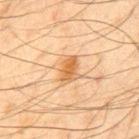| feature | finding |
|---|---|
| follow-up | no biopsy performed (imaged during a skin exam) |
| illumination | cross-polarized illumination |
| patient | male, aged approximately 45 |
| image-analysis metrics | border irregularity of about 3 on a 0–10 scale; a nevus-likeness score of about 90/100 and a detector confidence of about 100 out of 100 that the crop contains a lesion |
| body site | the mid back |
| acquisition | ~15 mm tile from a whole-body skin photo |